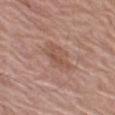workup = imaged on a skin check; not biopsied